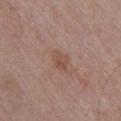Part of a total-body skin-imaging series; this lesion was reviewed on a skin check and was not flagged for biopsy.
Located on the chest.
The lesion-visualizer software estimated a classifier nevus-likeness of about 0/100.
A female patient approximately 50 years of age.
Measured at roughly 3 mm in maximum diameter.
A lesion tile, about 15 mm wide, cut from a 3D total-body photograph.